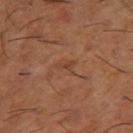The lesion's longest dimension is about 3 mm.
A 15 mm close-up tile from a total-body photography series done for melanoma screening.
Imaged with cross-polarized lighting.
A male patient, approximately 65 years of age.
Located on the right thigh.